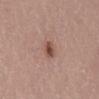Impression:
Part of a total-body skin-imaging series; this lesion was reviewed on a skin check and was not flagged for biopsy.
Image and clinical context:
A female subject in their 70s. A 15 mm close-up tile from a total-body photography series done for melanoma screening. The lesion is located on the mid back.